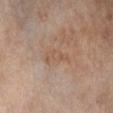Recorded during total-body skin imaging; not selected for excision or biopsy. The tile uses cross-polarized illumination. Approximately 4 mm at its widest. From the right lower leg. A 15 mm close-up extracted from a 3D total-body photography capture. The patient is a female aged approximately 50.